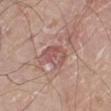Notes:
- notes: catalogued during a skin exam; not biopsied